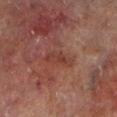Imaged during a routine full-body skin examination; the lesion was not biopsied and no histopathology is available. From the leg. This is a cross-polarized tile. Cropped from a whole-body photographic skin survey; the tile spans about 15 mm. About 3.5 mm across. A male patient aged 68 to 72.